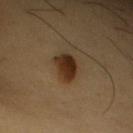Impression: Captured during whole-body skin photography for melanoma surveillance; the lesion was not biopsied. Background: A 15 mm close-up tile from a total-body photography series done for melanoma screening. Measured at roughly 3.5 mm in maximum diameter. This is a cross-polarized tile. The subject is a female aged 53–57. Located on the right upper arm.